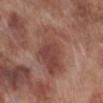Notes:
* automated metrics · a lesion color around L≈45 a*≈22 b*≈25 in CIELAB; a border-irregularity index near 3.5/10 and radial color variation of about 2
* patient · male, aged 68 to 72
* site · the right lower leg
* tile lighting · white-light illumination
* size · about 6.5 mm
* imaging modality · total-body-photography crop, ~15 mm field of view